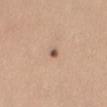From the mid back. This image is a 15 mm lesion crop taken from a total-body photograph. A female patient aged approximately 35. Measured at roughly 1.5 mm in maximum diameter. Captured under white-light illumination. The lesion-visualizer software estimated a shape eccentricity near 0.5 and a shape-asymmetry score of about 0.25 (0 = symmetric). The analysis additionally found border irregularity of about 2 on a 0–10 scale, a within-lesion color-variation index near 0/10, and a peripheral color-asymmetry measure near 0. The software also gave a nevus-likeness score of about 95/100 and lesion-presence confidence of about 100/100.